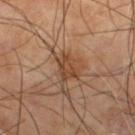{"biopsy_status": "not biopsied; imaged during a skin examination", "lesion_size": {"long_diameter_mm_approx": 3.5}, "image": {"source": "total-body photography crop", "field_of_view_mm": 15}, "patient": {"sex": "male", "age_approx": 70}, "lighting": "cross-polarized", "site": "left lower leg"}